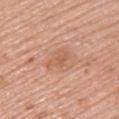Captured during whole-body skin photography for melanoma surveillance; the lesion was not biopsied.
The lesion is on the upper back.
A region of skin cropped from a whole-body photographic capture, roughly 15 mm wide.
The tile uses white-light illumination.
The lesion-visualizer software estimated a border-irregularity index near 4.5/10, a color-variation rating of about 1/10, and peripheral color asymmetry of about 0. And it measured an automated nevus-likeness rating near 0 out of 100 and lesion-presence confidence of about 100/100.
A female subject aged 58–62.
Measured at roughly 3 mm in maximum diameter.